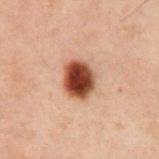workup: total-body-photography surveillance lesion; no biopsy
subject: male, about 60 years old
illumination: cross-polarized
diameter: ~4 mm (longest diameter)
anatomic site: the abdomen
image: 15 mm crop, total-body photography